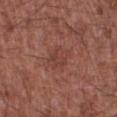imaging modality: 15 mm crop, total-body photography | body site: the right lower leg | subject: male, aged 63–67 | size: ~3 mm (longest diameter) | image-analysis metrics: an average lesion color of about L≈41 a*≈24 b*≈25 (CIELAB), a lesion–skin lightness drop of about 6, and a lesion-to-skin contrast of about 4.5 (normalized; higher = more distinct); a within-lesion color-variation index near 2.5/10 and peripheral color asymmetry of about 1.